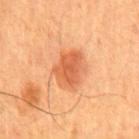The lesion was photographed on a routine skin check and not biopsied; there is no pathology result. A male patient in their mid- to late 50s. Cropped from a total-body skin-imaging series; the visible field is about 15 mm. The lesion is on the chest.